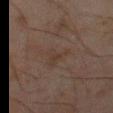A 15 mm close-up extracted from a 3D total-body photography capture. Approximately 3.5 mm at its widest. This is a cross-polarized tile. Located on the left thigh. A male subject, roughly 45 years of age. The lesion-visualizer software estimated a lesion area of about 4.5 mm², an eccentricity of roughly 0.8, and two-axis asymmetry of about 0.6. It also reported a border-irregularity index near 6.5/10, a color-variation rating of about 1/10, and peripheral color asymmetry of about 0.5. The analysis additionally found an automated nevus-likeness rating near 0 out of 100.